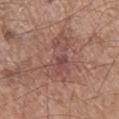notes: total-body-photography surveillance lesion; no biopsy
imaging modality: 15 mm crop, total-body photography
site: the right lower leg
subject: male, aged 58 to 62